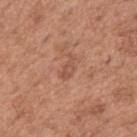The lesion was tiled from a total-body skin photograph and was not biopsied.
A 15 mm close-up extracted from a 3D total-body photography capture.
The lesion is located on the upper back.
A male patient, aged 63–67.